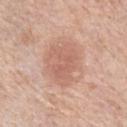biopsy status: total-body-photography surveillance lesion; no biopsy | body site: the left upper arm | image: ~15 mm crop, total-body skin-cancer survey | tile lighting: white-light illumination | automated metrics: a lesion color around L≈62 a*≈22 b*≈28 in CIELAB and a normalized border contrast of about 5; a color-variation rating of about 3.5/10 and radial color variation of about 1 | patient: female, about 65 years old.